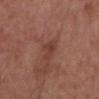acquisition — 15 mm crop, total-body photography | anatomic site — the head or neck | subject — male, aged approximately 55 | size — ≈3.5 mm.